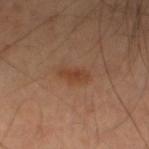<record>
<biopsy_status>not biopsied; imaged during a skin examination</biopsy_status>
<patient>
  <sex>male</sex>
  <age_approx>40</age_approx>
</patient>
<site>left lower leg</site>
<image>
  <source>total-body photography crop</source>
  <field_of_view_mm>15</field_of_view_mm>
</image>
<automated_metrics>
  <eccentricity>0.85</eccentricity>
  <shape_asymmetry>0.3</shape_asymmetry>
  <cielab_L>41</cielab_L>
  <cielab_a>21</cielab_a>
  <cielab_b>32</cielab_b>
  <vs_skin_contrast_norm>6.5</vs_skin_contrast_norm>
  <border_irregularity_0_10>3.0</border_irregularity_0_10>
  <color_variation_0_10>1.5</color_variation_0_10>
  <peripheral_color_asymmetry>0.5</peripheral_color_asymmetry>
</automated_metrics>
<lighting>cross-polarized</lighting>
</record>